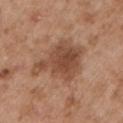This lesion was catalogued during total-body skin photography and was not selected for biopsy. The tile uses white-light illumination. A male patient approximately 55 years of age. A 15 mm crop from a total-body photograph taken for skin-cancer surveillance. Longest diameter approximately 7 mm. Located on the left upper arm. An algorithmic analysis of the crop reported an area of roughly 19 mm², a shape eccentricity near 0.7, and a shape-asymmetry score of about 0.45 (0 = symmetric). The analysis additionally found a lesion color around L≈48 a*≈21 b*≈31 in CIELAB, about 11 CIELAB-L* units darker than the surrounding skin, and a normalized lesion–skin contrast near 8. And it measured a classifier nevus-likeness of about 20/100 and lesion-presence confidence of about 100/100.